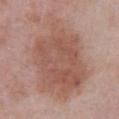Imaged during a routine full-body skin examination; the lesion was not biopsied and no histopathology is available.
About 11.5 mm across.
The lesion-visualizer software estimated an area of roughly 60 mm², an outline eccentricity of about 0.75 (0 = round, 1 = elongated), and a symmetry-axis asymmetry near 0.2. The software also gave a mean CIELAB color near L≈54 a*≈20 b*≈27, about 9 CIELAB-L* units darker than the surrounding skin, and a normalized lesion–skin contrast near 7.
The subject is a male in their mid- to late 50s.
A 15 mm close-up tile from a total-body photography series done for melanoma screening.
From the front of the torso.